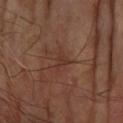Q: Is there a histopathology result?
A: total-body-photography surveillance lesion; no biopsy
Q: Who is the patient?
A: male, aged around 70
Q: Where on the body is the lesion?
A: the right forearm
Q: How was this image acquired?
A: ~15 mm crop, total-body skin-cancer survey
Q: Automated lesion metrics?
A: a mean CIELAB color near L≈33 a*≈20 b*≈24, about 5 CIELAB-L* units darker than the surrounding skin, and a normalized border contrast of about 5; a classifier nevus-likeness of about 0/100 and lesion-presence confidence of about 95/100